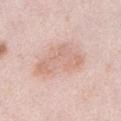Q: Was this lesion biopsied?
A: catalogued during a skin exam; not biopsied
Q: What is the anatomic site?
A: the abdomen
Q: What is the imaging modality?
A: total-body-photography crop, ~15 mm field of view
Q: How was the tile lit?
A: white-light illumination
Q: What did automated image analysis measure?
A: a lesion area of about 21 mm², an eccentricity of roughly 0.8, and a shape-asymmetry score of about 0.25 (0 = symmetric); a lesion color around L≈69 a*≈19 b*≈26 in CIELAB, a lesion–skin lightness drop of about 8, and a lesion-to-skin contrast of about 5 (normalized; higher = more distinct)
Q: Who is the patient?
A: female, about 65 years old
Q: How large is the lesion?
A: ≈7 mm